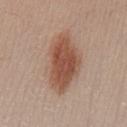The lesion was tiled from a total-body skin photograph and was not biopsied.
The lesion's longest dimension is about 7 mm.
Located on the left lower leg.
Captured under white-light illumination.
The subject is a female aged around 25.
Cropped from a whole-body photographic skin survey; the tile spans about 15 mm.
Automated tile analysis of the lesion measured a lesion–skin lightness drop of about 13 and a lesion-to-skin contrast of about 9.5 (normalized; higher = more distinct). And it measured a border-irregularity rating of about 2.5/10, internal color variation of about 4 on a 0–10 scale, and radial color variation of about 1. It also reported an automated nevus-likeness rating near 100 out of 100 and lesion-presence confidence of about 100/100.